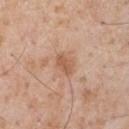workup: imaged on a skin check; not biopsied
location: the chest
size: ≈2.5 mm
acquisition: total-body-photography crop, ~15 mm field of view
lighting: white-light
automated lesion analysis: a lesion color around L≈57 a*≈22 b*≈33 in CIELAB, roughly 9 lightness units darker than nearby skin, and a lesion-to-skin contrast of about 6.5 (normalized; higher = more distinct); border irregularity of about 2 on a 0–10 scale, a within-lesion color-variation index near 1.5/10, and peripheral color asymmetry of about 0.5
patient: male, aged 58 to 62